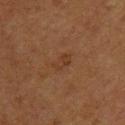On the upper back. The tile uses cross-polarized illumination. Approximately 2.5 mm at its widest. A female patient, aged approximately 50. This image is a 15 mm lesion crop taken from a total-body photograph. Automated tile analysis of the lesion measured an eccentricity of roughly 0.85 and two-axis asymmetry of about 0.3. The software also gave a lesion color around L≈31 a*≈18 b*≈27 in CIELAB, a lesion–skin lightness drop of about 5, and a lesion-to-skin contrast of about 5 (normalized; higher = more distinct).